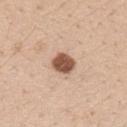Q: Is there a histopathology result?
A: imaged on a skin check; not biopsied
Q: What kind of image is this?
A: ~15 mm crop, total-body skin-cancer survey
Q: What is the lesion's diameter?
A: ≈3 mm
Q: What lighting was used for the tile?
A: white-light illumination
Q: Patient demographics?
A: female, in their mid- to late 20s
Q: What is the anatomic site?
A: the left forearm